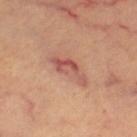Recorded during total-body skin imaging; not selected for excision or biopsy. This image is a 15 mm lesion crop taken from a total-body photograph. On the left thigh. A subject approximately 60 years of age. Longest diameter approximately 5 mm.